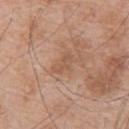| key | value |
|---|---|
| biopsy status | imaged on a skin check; not biopsied |
| patient | male, in their mid-60s |
| location | the upper back |
| image | ~15 mm crop, total-body skin-cancer survey |